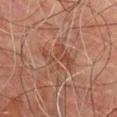The lesion was tiled from a total-body skin photograph and was not biopsied. Captured under cross-polarized illumination. The lesion is on the chest. A 15 mm close-up extracted from a 3D total-body photography capture. Longest diameter approximately 4.5 mm. A male patient aged 73–77.